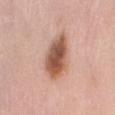image source: ~15 mm tile from a whole-body skin photo; patient: female, about 50 years old; anatomic site: the back.